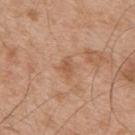Part of a total-body skin-imaging series; this lesion was reviewed on a skin check and was not flagged for biopsy. A male patient roughly 55 years of age. A 15 mm close-up tile from a total-body photography series done for melanoma screening. The lesion is on the upper back.